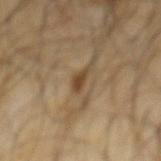Captured during whole-body skin photography for melanoma surveillance; the lesion was not biopsied.
A male subject roughly 65 years of age.
Cropped from a whole-body photographic skin survey; the tile spans about 15 mm.
The lesion is on the back.
Captured under cross-polarized illumination.